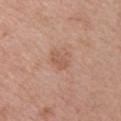Captured during whole-body skin photography for melanoma surveillance; the lesion was not biopsied.
The lesion is located on the front of the torso.
A roughly 15 mm field-of-view crop from a total-body skin photograph.
Imaged with white-light lighting.
The patient is a male roughly 60 years of age.